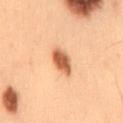notes: catalogued during a skin exam; not biopsied | patient: male, aged 53 to 57 | automated metrics: a footprint of about 6 mm² and a shape-asymmetry score of about 0.2 (0 = symmetric); about 15 CIELAB-L* units darker than the surrounding skin; radial color variation of about 1 | imaging modality: ~15 mm crop, total-body skin-cancer survey | illumination: cross-polarized illumination | location: the mid back | lesion size: about 3.5 mm.